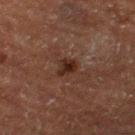workup = catalogued during a skin exam; not biopsied | automated metrics = a lesion area of about 4 mm² and a shape eccentricity near 0.55; a lesion color around L≈21 a*≈16 b*≈20 in CIELAB, a lesion–skin lightness drop of about 8, and a normalized border contrast of about 9; a border-irregularity rating of about 3/10 and peripheral color asymmetry of about 1.5; an automated nevus-likeness rating near 75 out of 100 and a lesion-detection confidence of about 100/100 | tile lighting = cross-polarized illumination | imaging modality = total-body-photography crop, ~15 mm field of view | location = the left thigh | patient = male, aged around 75.